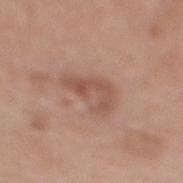Assessment:
Captured during whole-body skin photography for melanoma surveillance; the lesion was not biopsied.
Acquisition and patient details:
The lesion is on the mid back. A 15 mm close-up extracted from a 3D total-body photography capture. Imaged with white-light lighting. A female subject aged 68–72.